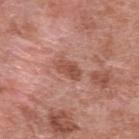Q: Was a biopsy performed?
A: catalogued during a skin exam; not biopsied
Q: Who is the patient?
A: female, aged 68 to 72
Q: What lighting was used for the tile?
A: white-light
Q: Lesion location?
A: the upper back
Q: How was this image acquired?
A: total-body-photography crop, ~15 mm field of view
Q: Automated lesion metrics?
A: a footprint of about 5 mm² and an eccentricity of roughly 0.85; roughly 10 lightness units darker than nearby skin and a lesion-to-skin contrast of about 7.5 (normalized; higher = more distinct); a nevus-likeness score of about 0/100 and lesion-presence confidence of about 100/100